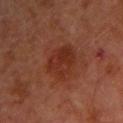The lesion was photographed on a routine skin check and not biopsied; there is no pathology result.
Imaged with cross-polarized lighting.
This image is a 15 mm lesion crop taken from a total-body photograph.
The recorded lesion diameter is about 4 mm.
From the right upper arm.
The subject is a male roughly 65 years of age.
The total-body-photography lesion software estimated an area of roughly 11 mm², an eccentricity of roughly 0.4, and a symmetry-axis asymmetry near 0.25. The analysis additionally found a mean CIELAB color near L≈27 a*≈24 b*≈27, about 6 CIELAB-L* units darker than the surrounding skin, and a normalized border contrast of about 6.5. The analysis additionally found an automated nevus-likeness rating near 30 out of 100 and a lesion-detection confidence of about 100/100.